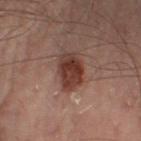Impression: Recorded during total-body skin imaging; not selected for excision or biopsy. Acquisition and patient details: The recorded lesion diameter is about 3.5 mm. The subject is a male approximately 50 years of age. Automated image analysis of the tile measured an eccentricity of roughly 0.6 and a shape-asymmetry score of about 0.15 (0 = symmetric). And it measured an automated nevus-likeness rating near 95 out of 100. A 15 mm close-up tile from a total-body photography series done for melanoma screening. This is a cross-polarized tile. The lesion is on the left leg.